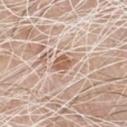biopsy status: catalogued during a skin exam; not biopsied | patient: male, aged 78–82 | image: ~15 mm tile from a whole-body skin photo | location: the chest.